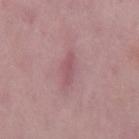Findings:
* workup: imaged on a skin check; not biopsied
* lighting: white-light illumination
* patient: male, aged 48 to 52
* acquisition: total-body-photography crop, ~15 mm field of view
* TBP lesion metrics: a footprint of about 2.5 mm², an outline eccentricity of about 0.95 (0 = round, 1 = elongated), and two-axis asymmetry of about 0.4; a lesion color around L≈52 a*≈25 b*≈16 in CIELAB and a lesion–skin lightness drop of about 8; a lesion-detection confidence of about 65/100
* size: ~3 mm (longest diameter)
* site: the mid back